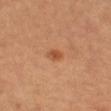Recorded during total-body skin imaging; not selected for excision or biopsy.
Automated image analysis of the tile measured a lesion color around L≈50 a*≈26 b*≈37 in CIELAB and about 9 CIELAB-L* units darker than the surrounding skin.
The subject is roughly 70 years of age.
The lesion is located on the left thigh.
Cropped from a whole-body photographic skin survey; the tile spans about 15 mm.